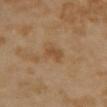{"biopsy_status": "not biopsied; imaged during a skin examination", "patient": {"sex": "female", "age_approx": 35}, "image": {"source": "total-body photography crop", "field_of_view_mm": 15}, "lesion_size": {"long_diameter_mm_approx": 3.0}, "automated_metrics": {"eccentricity": 0.75, "shape_asymmetry": 0.25, "border_irregularity_0_10": 3.0, "color_variation_0_10": 1.5, "peripheral_color_asymmetry": 0.5}, "site": "chest"}